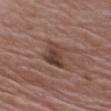– location: the chest
– lesion size: about 3.5 mm
– image source: 15 mm crop, total-body photography
– subject: male, aged 63–67